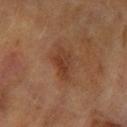Q: Was this lesion biopsied?
A: no biopsy performed (imaged during a skin exam)
Q: What did automated image analysis measure?
A: a shape eccentricity near 0.85 and a shape-asymmetry score of about 0.25 (0 = symmetric); a lesion color around L≈35 a*≈20 b*≈29 in CIELAB, a lesion–skin lightness drop of about 7, and a normalized border contrast of about 6.5; a classifier nevus-likeness of about 25/100 and a lesion-detection confidence of about 100/100
Q: What is the anatomic site?
A: the left forearm
Q: Illumination type?
A: cross-polarized illumination
Q: Patient demographics?
A: female, aged 58–62
Q: What kind of image is this?
A: total-body-photography crop, ~15 mm field of view